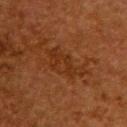follow-up=catalogued during a skin exam; not biopsied
anatomic site=the upper back
diameter=≈5.5 mm
subject=female, aged approximately 50
lighting=cross-polarized
image=~15 mm tile from a whole-body skin photo